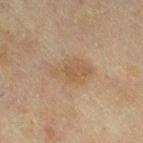Background: The subject is a female approximately 55 years of age. Automated tile analysis of the lesion measured an area of roughly 11 mm², an outline eccentricity of about 0.8 (0 = round, 1 = elongated), and two-axis asymmetry of about 0.2. And it measured an average lesion color of about L≈48 a*≈14 b*≈29 (CIELAB) and about 6 CIELAB-L* units darker than the surrounding skin. The analysis additionally found an automated nevus-likeness rating near 0 out of 100 and a lesion-detection confidence of about 100/100. Imaged with cross-polarized lighting. The lesion is located on the right thigh. The lesion's longest dimension is about 5 mm. This image is a 15 mm lesion crop taken from a total-body photograph.